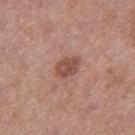biopsy status = no biopsy performed (imaged during a skin exam); body site = the right thigh; subject = female, aged approximately 40; lighting = white-light illumination; diameter = about 3.5 mm; imaging modality = total-body-photography crop, ~15 mm field of view.